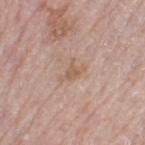workup: no biopsy performed (imaged during a skin exam); subject: female, aged approximately 65; image-analysis metrics: a border-irregularity index near 5.5/10 and a color-variation rating of about 1/10; imaging modality: total-body-photography crop, ~15 mm field of view; location: the left thigh; lesion size: ≈3 mm.